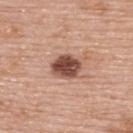Clinical summary: Located on the back. Imaged with white-light lighting. A close-up tile cropped from a whole-body skin photograph, about 15 mm across. The patient is a female aged approximately 60.